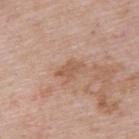Captured during whole-body skin photography for melanoma surveillance; the lesion was not biopsied. Automated tile analysis of the lesion measured a border-irregularity rating of about 3.5/10, a color-variation rating of about 1/10, and peripheral color asymmetry of about 0.5. A roughly 15 mm field-of-view crop from a total-body skin photograph. Longest diameter approximately 3 mm. On the upper back. This is a white-light tile. A male subject, about 65 years old.